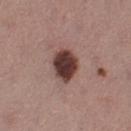Q: Is there a histopathology result?
A: no biopsy performed (imaged during a skin exam)
Q: Automated lesion metrics?
A: a lesion area of about 9.5 mm², a shape eccentricity near 0.6, and a symmetry-axis asymmetry near 0.2; a nevus-likeness score of about 70/100 and a lesion-detection confidence of about 100/100
Q: What is the lesion's diameter?
A: ~4 mm (longest diameter)
Q: Lesion location?
A: the left thigh
Q: Illumination type?
A: white-light illumination
Q: What kind of image is this?
A: ~15 mm tile from a whole-body skin photo
Q: Who is the patient?
A: female, in their mid-50s